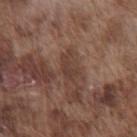• workup · imaged on a skin check; not biopsied
• imaging modality · ~15 mm tile from a whole-body skin photo
• diameter · about 2.5 mm
• subject · male, in their mid-70s
• tile lighting · white-light
• anatomic site · the chest